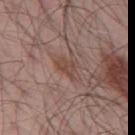Impression:
No biopsy was performed on this lesion — it was imaged during a full skin examination and was not determined to be concerning.
Background:
From the left thigh. The tile uses white-light illumination. A 15 mm close-up tile from a total-body photography series done for melanoma screening. The lesion's longest dimension is about 3 mm. A male patient aged approximately 55.